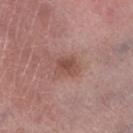Assessment: Recorded during total-body skin imaging; not selected for excision or biopsy. Clinical summary: Imaged with white-light lighting. The lesion is located on the left thigh. Measured at roughly 3 mm in maximum diameter. A female subject aged 68 to 72. A 15 mm crop from a total-body photograph taken for skin-cancer surveillance.